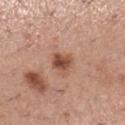Assessment: Part of a total-body skin-imaging series; this lesion was reviewed on a skin check and was not flagged for biopsy. Acquisition and patient details: On the right lower leg. Measured at roughly 2.5 mm in maximum diameter. A region of skin cropped from a whole-body photographic capture, roughly 15 mm wide. This is a white-light tile. A female subject, aged approximately 30. An algorithmic analysis of the crop reported an area of roughly 5 mm² and a shape eccentricity near 0.35. The software also gave about 13 CIELAB-L* units darker than the surrounding skin and a normalized lesion–skin contrast near 9.